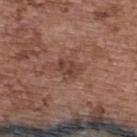No biopsy was performed on this lesion — it was imaged during a full skin examination and was not determined to be concerning. A female subject, in their mid-60s. On the upper back. Measured at roughly 3 mm in maximum diameter. A 15 mm close-up tile from a total-body photography series done for melanoma screening. Automated tile analysis of the lesion measured a lesion color around L≈41 a*≈21 b*≈25 in CIELAB and about 8 CIELAB-L* units darker than the surrounding skin. Captured under white-light illumination.